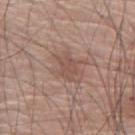A male subject, about 75 years old. A roughly 15 mm field-of-view crop from a total-body skin photograph. Imaged with white-light lighting. The lesion is on the right upper arm. Approximately 3 mm at its widest.